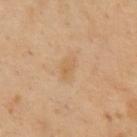Clinical impression: The lesion was photographed on a routine skin check and not biopsied; there is no pathology result. Acquisition and patient details: On the chest. A male patient, aged approximately 50. This image is a 15 mm lesion crop taken from a total-body photograph. Imaged with cross-polarized lighting.